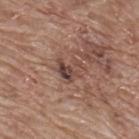Case summary:
- notes · no biopsy performed (imaged during a skin exam)
- imaging modality · total-body-photography crop, ~15 mm field of view
- location · the upper back
- size · ~3 mm (longest diameter)
- subject · male, in their 70s
- automated lesion analysis · a nevus-likeness score of about 25/100 and a lesion-detection confidence of about 90/100